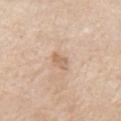Background: A 15 mm close-up tile from a total-body photography series done for melanoma screening. About 2.5 mm across. This is a white-light tile. A male subject, roughly 85 years of age or older. From the left upper arm.